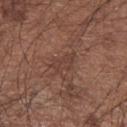{
  "image": {
    "source": "total-body photography crop",
    "field_of_view_mm": 15
  },
  "lighting": "white-light",
  "patient": {
    "sex": "male",
    "age_approx": 65
  },
  "lesion_size": {
    "long_diameter_mm_approx": 3.0
  },
  "site": "left upper arm"
}